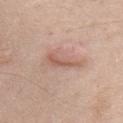Recorded during total-body skin imaging; not selected for excision or biopsy.
A male patient, about 70 years old.
A 15 mm crop from a total-body photograph taken for skin-cancer surveillance.
On the upper back.